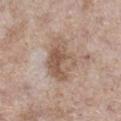– workup — total-body-photography surveillance lesion; no biopsy
– subject — female, aged 53 to 57
– body site — the right lower leg
– acquisition — total-body-photography crop, ~15 mm field of view
– size — ~5 mm (longest diameter)
– automated lesion analysis — a lesion color around L≈56 a*≈16 b*≈27 in CIELAB, roughly 10 lightness units darker than nearby skin, and a lesion-to-skin contrast of about 7 (normalized; higher = more distinct); an automated nevus-likeness rating near 0 out of 100 and a lesion-detection confidence of about 100/100